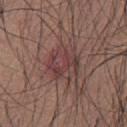{"biopsy_status": "not biopsied; imaged during a skin examination", "lighting": "white-light", "image": {"source": "total-body photography crop", "field_of_view_mm": 15}, "site": "chest", "patient": {"sex": "male", "age_approx": 35}}